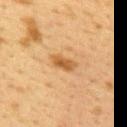Q: Was this lesion biopsied?
A: total-body-photography surveillance lesion; no biopsy
Q: Who is the patient?
A: female, roughly 40 years of age
Q: What is the imaging modality?
A: ~15 mm tile from a whole-body skin photo
Q: Automated lesion metrics?
A: a shape-asymmetry score of about 0.3 (0 = symmetric); a lesion color around L≈48 a*≈19 b*≈37 in CIELAB, about 10 CIELAB-L* units darker than the surrounding skin, and a lesion-to-skin contrast of about 8 (normalized; higher = more distinct)
Q: Where on the body is the lesion?
A: the upper back
Q: How large is the lesion?
A: ~3.5 mm (longest diameter)
Q: Illumination type?
A: cross-polarized illumination